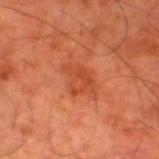Captured during whole-body skin photography for melanoma surveillance; the lesion was not biopsied.
The tile uses cross-polarized illumination.
The lesion is located on the leg.
A male patient, about 80 years old.
Measured at roughly 4 mm in maximum diameter.
Cropped from a total-body skin-imaging series; the visible field is about 15 mm.
An algorithmic analysis of the crop reported an area of roughly 7 mm², an eccentricity of roughly 0.75, and a shape-asymmetry score of about 0.45 (0 = symmetric). And it measured a border-irregularity rating of about 5/10. And it measured a nevus-likeness score of about 5/100 and a detector confidence of about 100 out of 100 that the crop contains a lesion.